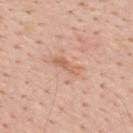Clinical impression: No biopsy was performed on this lesion — it was imaged during a full skin examination and was not determined to be concerning. Image and clinical context: The lesion is on the upper back. A male patient, approximately 55 years of age. A close-up tile cropped from a whole-body skin photograph, about 15 mm across.